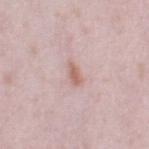workup: catalogued during a skin exam; not biopsied
patient: female, approximately 40 years of age
automated metrics: a footprint of about 3 mm², a shape eccentricity near 0.9, and a shape-asymmetry score of about 0.3 (0 = symmetric); a lesion color around L≈63 a*≈20 b*≈24 in CIELAB, a lesion–skin lightness drop of about 10, and a lesion-to-skin contrast of about 7 (normalized; higher = more distinct); a border-irregularity rating of about 3/10, a color-variation rating of about 2.5/10, and a peripheral color-asymmetry measure near 1; a nevus-likeness score of about 100/100 and lesion-presence confidence of about 100/100
site: the right thigh
imaging modality: ~15 mm crop, total-body skin-cancer survey